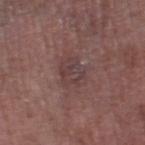Imaged with white-light lighting. From the leg. A male patient, aged 73 to 77. This image is a 15 mm lesion crop taken from a total-body photograph. Approximately 3.5 mm at its widest.